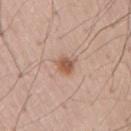Captured during whole-body skin photography for melanoma surveillance; the lesion was not biopsied.
A male subject, about 55 years old.
An algorithmic analysis of the crop reported a lesion area of about 4.5 mm², a shape eccentricity near 0.6, and a symmetry-axis asymmetry near 0.25. It also reported a mean CIELAB color near L≈56 a*≈21 b*≈30, a lesion–skin lightness drop of about 11, and a normalized border contrast of about 8. The analysis additionally found border irregularity of about 2 on a 0–10 scale, a color-variation rating of about 3.5/10, and peripheral color asymmetry of about 1.5. It also reported a nevus-likeness score of about 95/100 and a lesion-detection confidence of about 100/100.
The recorded lesion diameter is about 2.5 mm.
Captured under white-light illumination.
Cropped from a total-body skin-imaging series; the visible field is about 15 mm.